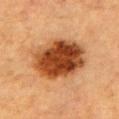biopsy_status: not biopsied; imaged during a skin examination
lighting: cross-polarized
patient:
  sex: male
  age_approx: 85+
image:
  source: total-body photography crop
  field_of_view_mm: 15
site: chest